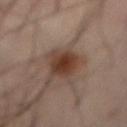Part of a total-body skin-imaging series; this lesion was reviewed on a skin check and was not flagged for biopsy. The subject is a male in their mid- to late 60s. This is a cross-polarized tile. The lesion's longest dimension is about 4.5 mm. On the front of the torso. A close-up tile cropped from a whole-body skin photograph, about 15 mm across. The total-body-photography lesion software estimated a lesion color around L≈35 a*≈17 b*≈25 in CIELAB and a lesion-to-skin contrast of about 11 (normalized; higher = more distinct). The software also gave a border-irregularity rating of about 3.5/10, a color-variation rating of about 3.5/10, and a peripheral color-asymmetry measure near 1.